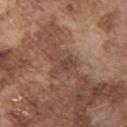{
  "biopsy_status": "not biopsied; imaged during a skin examination",
  "image": {
    "source": "total-body photography crop",
    "field_of_view_mm": 15
  },
  "patient": {
    "sex": "male",
    "age_approx": 75
  },
  "site": "chest",
  "automated_metrics": {
    "area_mm2_approx": 6.0,
    "eccentricity": 0.7,
    "shape_asymmetry": 0.55,
    "cielab_L": 44,
    "cielab_a": 19,
    "cielab_b": 27,
    "vs_skin_darker_L": 7.0,
    "nevus_likeness_0_100": 0,
    "lesion_detection_confidence_0_100": 75
  },
  "lesion_size": {
    "long_diameter_mm_approx": 3.5
  }
}